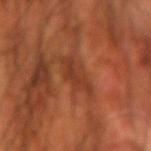Clinical impression: Recorded during total-body skin imaging; not selected for excision or biopsy.